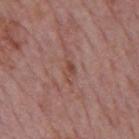| field | value |
|---|---|
| biopsy status | no biopsy performed (imaged during a skin exam) |
| lesion size | about 3.5 mm |
| location | the back |
| image | 15 mm crop, total-body photography |
| subject | male, approximately 75 years of age |
| tile lighting | white-light |